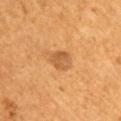<lesion>
  <biopsy_status>not biopsied; imaged during a skin examination</biopsy_status>
  <site>arm</site>
  <image>
    <source>total-body photography crop</source>
    <field_of_view_mm>15</field_of_view_mm>
  </image>
  <patient>
    <sex>male</sex>
    <age_approx>55</age_approx>
  </patient>
  <lesion_size>
    <long_diameter_mm_approx>2.5</long_diameter_mm_approx>
  </lesion_size>
</lesion>